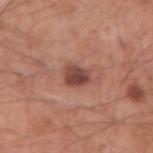Clinical impression:
Captured during whole-body skin photography for melanoma surveillance; the lesion was not biopsied.
Acquisition and patient details:
A 15 mm close-up tile from a total-body photography series done for melanoma screening. Imaged with white-light lighting. The subject is a male aged around 60. Located on the right upper arm. The recorded lesion diameter is about 2.5 mm.